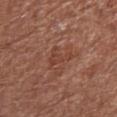Notes:
* workup: imaged on a skin check; not biopsied
* imaging modality: total-body-photography crop, ~15 mm field of view
* site: the right upper arm
* subject: female, aged approximately 80
* automated metrics: a shape eccentricity near 0.9 and two-axis asymmetry of about 0.55; a border-irregularity index near 6.5/10, a color-variation rating of about 1.5/10, and radial color variation of about 0; an automated nevus-likeness rating near 0 out of 100 and a lesion-detection confidence of about 100/100
* lesion diameter: about 3.5 mm